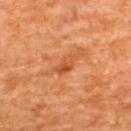notes = imaged on a skin check; not biopsied
lighting = cross-polarized
imaging modality = 15 mm crop, total-body photography
size = ≈3 mm
site = the upper back
patient = male, aged approximately 65